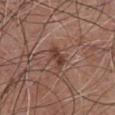workup: imaged on a skin check; not biopsied
automated metrics: a footprint of about 4.5 mm², an eccentricity of roughly 0.85, and a shape-asymmetry score of about 0.25 (0 = symmetric); an automated nevus-likeness rating near 40 out of 100 and a lesion-detection confidence of about 100/100
lighting: white-light illumination
imaging modality: 15 mm crop, total-body photography
subject: male, in their mid-70s
diameter: ~3 mm (longest diameter)
site: the chest